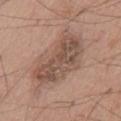Findings:
• follow-up: total-body-photography surveillance lesion; no biopsy
• automated lesion analysis: an automated nevus-likeness rating near 5 out of 100 and lesion-presence confidence of about 100/100
• lesion diameter: ~7.5 mm (longest diameter)
• subject: male, about 55 years old
• image: ~15 mm tile from a whole-body skin photo
• anatomic site: the front of the torso